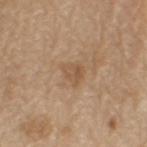  biopsy_status: not biopsied; imaged during a skin examination
  image:
    source: total-body photography crop
    field_of_view_mm: 15
  site: left upper arm
  patient:
    sex: male
    age_approx: 70